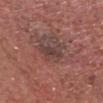notes = total-body-photography surveillance lesion; no biopsy | lesion diameter = about 3.5 mm | lighting = white-light | location = the head or neck | subject = male, roughly 70 years of age | image source = ~15 mm tile from a whole-body skin photo.